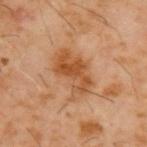Imaged during a routine full-body skin examination; the lesion was not biopsied and no histopathology is available. A male patient, aged approximately 60. The lesion is on the upper back. Longest diameter approximately 5.5 mm. A 15 mm crop from a total-body photograph taken for skin-cancer surveillance. This is a cross-polarized tile.